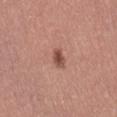{"biopsy_status": "not biopsied; imaged during a skin examination", "site": "mid back", "image": {"source": "total-body photography crop", "field_of_view_mm": 15}, "patient": {"sex": "female", "age_approx": 50}}